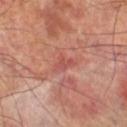Case summary:
• follow-up: no biopsy performed (imaged during a skin exam)
• image source: ~15 mm tile from a whole-body skin photo
• patient: male, about 70 years old
• anatomic site: the leg